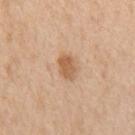Background: The patient is a male aged approximately 70. This is a white-light tile. A 15 mm close-up tile from a total-body photography series done for melanoma screening. On the chest.